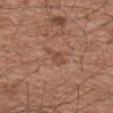No biopsy was performed on this lesion — it was imaged during a full skin examination and was not determined to be concerning.
A male subject about 75 years old.
The lesion-visualizer software estimated an average lesion color of about L≈48 a*≈22 b*≈29 (CIELAB) and a lesion-to-skin contrast of about 5.5 (normalized; higher = more distinct).
Captured under white-light illumination.
The lesion is on the upper back.
A region of skin cropped from a whole-body photographic capture, roughly 15 mm wide.